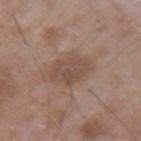{
  "biopsy_status": "not biopsied; imaged during a skin examination",
  "site": "left upper arm",
  "patient": {
    "sex": "male",
    "age_approx": 50
  },
  "image": {
    "source": "total-body photography crop",
    "field_of_view_mm": 15
  },
  "lighting": "white-light"
}